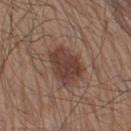workup — total-body-photography surveillance lesion; no biopsy
image — ~15 mm crop, total-body skin-cancer survey
illumination — white-light illumination
subject — male, roughly 60 years of age
location — the leg
lesion diameter — ≈4.5 mm